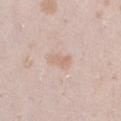Part of a total-body skin-imaging series; this lesion was reviewed on a skin check and was not flagged for biopsy. A roughly 15 mm field-of-view crop from a total-body skin photograph. The lesion is on the leg. A female patient in their mid-20s.